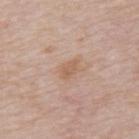No biopsy was performed on this lesion — it was imaged during a full skin examination and was not determined to be concerning.
A 15 mm close-up tile from a total-body photography series done for melanoma screening.
A male patient, aged approximately 75.
Located on the mid back.